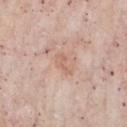Q: Was this lesion biopsied?
A: imaged on a skin check; not biopsied
Q: How was the tile lit?
A: white-light
Q: What is the imaging modality?
A: 15 mm crop, total-body photography
Q: Where on the body is the lesion?
A: the chest
Q: How large is the lesion?
A: ~2.5 mm (longest diameter)
Q: What did automated image analysis measure?
A: a border-irregularity rating of about 6.5/10 and a peripheral color-asymmetry measure near 0; a nevus-likeness score of about 0/100 and a detector confidence of about 100 out of 100 that the crop contains a lesion
Q: Who is the patient?
A: male, about 55 years old